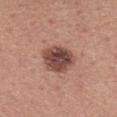The lesion was photographed on a routine skin check and not biopsied; there is no pathology result. The lesion is located on the mid back. A roughly 15 mm field-of-view crop from a total-body skin photograph. A male subject in their 40s.